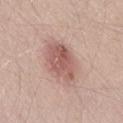Clinical impression: Part of a total-body skin-imaging series; this lesion was reviewed on a skin check and was not flagged for biopsy. Acquisition and patient details: About 5 mm across. From the right thigh. The tile uses white-light illumination. A lesion tile, about 15 mm wide, cut from a 3D total-body photograph. A male patient, approximately 40 years of age.